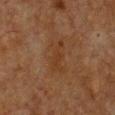follow-up — total-body-photography surveillance lesion; no biopsy | subject — female, aged around 55 | automated metrics — an automated nevus-likeness rating near 0 out of 100 | diameter — ≈4 mm | site — the chest | illumination — cross-polarized | imaging modality — ~15 mm crop, total-body skin-cancer survey.